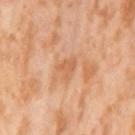The lesion was tiled from a total-body skin photograph and was not biopsied.
On the left thigh.
Cropped from a total-body skin-imaging series; the visible field is about 15 mm.
About 2.5 mm across.
A female subject aged 53 to 57.
An algorithmic analysis of the crop reported a lesion color around L≈62 a*≈25 b*≈38 in CIELAB, a lesion–skin lightness drop of about 8, and a normalized lesion–skin contrast near 5.5. The analysis additionally found a border-irregularity rating of about 4.5/10, internal color variation of about 1 on a 0–10 scale, and peripheral color asymmetry of about 0.5. The analysis additionally found a nevus-likeness score of about 0/100 and lesion-presence confidence of about 100/100.